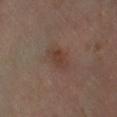A male patient about 70 years old.
An algorithmic analysis of the crop reported an area of roughly 6 mm² and a shape-asymmetry score of about 0.2 (0 = symmetric). The analysis additionally found roughly 6 lightness units darker than nearby skin and a normalized border contrast of about 6. It also reported a detector confidence of about 100 out of 100 that the crop contains a lesion.
A 15 mm crop from a total-body photograph taken for skin-cancer surveillance.
The lesion is on the left lower leg.